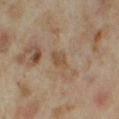Notes:
* workup — total-body-photography surveillance lesion; no biopsy
* illumination — cross-polarized illumination
* image — ~15 mm crop, total-body skin-cancer survey
* TBP lesion metrics — a lesion–skin lightness drop of about 7; a within-lesion color-variation index near 1.5/10 and peripheral color asymmetry of about 0.5
* body site — the right thigh
* patient — female, aged 33 to 37